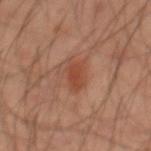Findings:
– follow-up — total-body-photography surveillance lesion; no biopsy
– image — 15 mm crop, total-body photography
– illumination — cross-polarized
– subject — male, aged 43 to 47
– TBP lesion metrics — radial color variation of about 0.5
– lesion diameter — ~3.5 mm (longest diameter)
– location — the mid back